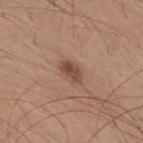Clinical impression: The lesion was tiled from a total-body skin photograph and was not biopsied. Acquisition and patient details: This image is a 15 mm lesion crop taken from a total-body photograph. Approximately 3 mm at its widest. A male patient aged approximately 30. The lesion is located on the upper back. Imaged with white-light lighting.